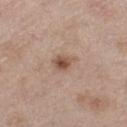The lesion was tiled from a total-body skin photograph and was not biopsied.
A female patient about 40 years old.
Measured at roughly 3 mm in maximum diameter.
The tile uses white-light illumination.
The lesion is located on the left thigh.
A 15 mm close-up tile from a total-body photography series done for melanoma screening.